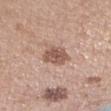The lesion was photographed on a routine skin check and not biopsied; there is no pathology result. A female patient, in their mid- to late 30s. On the right forearm. The total-body-photography lesion software estimated a lesion area of about 8 mm², an eccentricity of roughly 0.6, and a shape-asymmetry score of about 0.25 (0 = symmetric). The analysis additionally found a border-irregularity index near 2.5/10 and radial color variation of about 1.5. Cropped from a whole-body photographic skin survey; the tile spans about 15 mm. The lesion's longest dimension is about 3.5 mm.